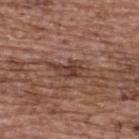{"lighting": "white-light", "patient": {"sex": "female", "age_approx": 65}, "site": "upper back", "image": {"source": "total-body photography crop", "field_of_view_mm": 15}, "lesion_size": {"long_diameter_mm_approx": 4.5}}